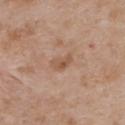Recorded during total-body skin imaging; not selected for excision or biopsy. The lesion is on the upper back. A female patient, aged approximately 30. The lesion's longest dimension is about 3 mm. The tile uses white-light illumination. A region of skin cropped from a whole-body photographic capture, roughly 15 mm wide.